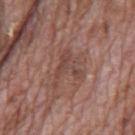notes: catalogued during a skin exam; not biopsied | patient: male, in their 70s | size: ≈5 mm | site: the mid back | illumination: white-light | image: ~15 mm crop, total-body skin-cancer survey.